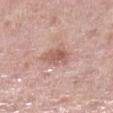workup: total-body-photography surveillance lesion; no biopsy
lighting: white-light
body site: the left lower leg
image: ~15 mm tile from a whole-body skin photo
diameter: about 3.5 mm
patient: female, approximately 50 years of age